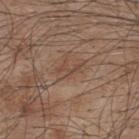Assessment: Captured during whole-body skin photography for melanoma surveillance; the lesion was not biopsied.